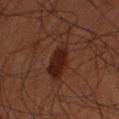Impression:
Recorded during total-body skin imaging; not selected for excision or biopsy.
Image and clinical context:
The patient is a male approximately 60 years of age. A 15 mm close-up tile from a total-body photography series done for melanoma screening. The tile uses cross-polarized illumination. The lesion is located on the right forearm.